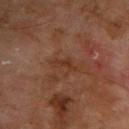Findings:
– biopsy status · catalogued during a skin exam; not biopsied
– size · ~4 mm (longest diameter)
– lighting · cross-polarized
– image source · ~15 mm tile from a whole-body skin photo
– patient · male, about 70 years old
– site · the upper back